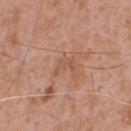| feature | finding |
|---|---|
| biopsy status | catalogued during a skin exam; not biopsied |
| lesion diameter | ~3 mm (longest diameter) |
| automated metrics | a footprint of about 3.5 mm² and an eccentricity of roughly 0.8; a mean CIELAB color near L≈54 a*≈22 b*≈32, a lesion–skin lightness drop of about 6, and a normalized border contrast of about 4.5; an automated nevus-likeness rating near 0 out of 100 |
| site | the chest |
| image | total-body-photography crop, ~15 mm field of view |
| subject | male, roughly 65 years of age |
| lighting | white-light illumination |